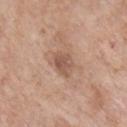{"biopsy_status": "not biopsied; imaged during a skin examination", "site": "front of the torso", "lighting": "white-light", "lesion_size": {"long_diameter_mm_approx": 3.5}, "patient": {"sex": "female", "age_approx": 60}, "image": {"source": "total-body photography crop", "field_of_view_mm": 15}}